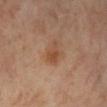Notes:
– workup — imaged on a skin check; not biopsied
– site — the right lower leg
– lighting — cross-polarized illumination
– automated metrics — a within-lesion color-variation index near 3/10 and a peripheral color-asymmetry measure near 1; an automated nevus-likeness rating near 40 out of 100 and lesion-presence confidence of about 100/100
– patient — female, aged 53–57
– diameter — ~3 mm (longest diameter)
– acquisition — ~15 mm tile from a whole-body skin photo